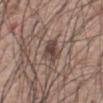Impression: This lesion was catalogued during total-body skin photography and was not selected for biopsy. Clinical summary: Captured under white-light illumination. Automated image analysis of the tile measured an automated nevus-likeness rating near 55 out of 100 and lesion-presence confidence of about 90/100. Cropped from a total-body skin-imaging series; the visible field is about 15 mm. From the abdomen. A male subject in their 60s. Longest diameter approximately 3.5 mm.